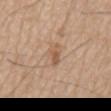{"biopsy_status": "not biopsied; imaged during a skin examination", "image": {"source": "total-body photography crop", "field_of_view_mm": 15}, "lighting": "white-light", "patient": {"sex": "male", "age_approx": 80}, "site": "mid back", "automated_metrics": {"cielab_L": 56, "cielab_a": 19, "cielab_b": 32, "vs_skin_darker_L": 9.0, "vs_skin_contrast_norm": 6.5, "border_irregularity_0_10": 4.5, "color_variation_0_10": 1.5, "peripheral_color_asymmetry": 0.5, "nevus_likeness_0_100": 5, "lesion_detection_confidence_0_100": 100}, "lesion_size": {"long_diameter_mm_approx": 3.0}}